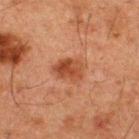workup: no biopsy performed (imaged during a skin exam)
automated lesion analysis: a lesion color around L≈37 a*≈23 b*≈29 in CIELAB, a lesion–skin lightness drop of about 9, and a normalized border contrast of about 8; a border-irregularity rating of about 2/10 and internal color variation of about 4.5 on a 0–10 scale
image: total-body-photography crop, ~15 mm field of view
illumination: cross-polarized illumination
anatomic site: the upper back
patient: male, in their 50s
lesion diameter: ≈3.5 mm